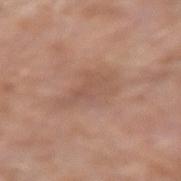No biopsy was performed on this lesion — it was imaged during a full skin examination and was not determined to be concerning.
On the arm.
Automated image analysis of the tile measured a shape eccentricity near 0.85 and a shape-asymmetry score of about 0.35 (0 = symmetric). And it measured border irregularity of about 4.5 on a 0–10 scale, internal color variation of about 3 on a 0–10 scale, and radial color variation of about 1. The software also gave a classifier nevus-likeness of about 0/100.
Imaged with white-light lighting.
A 15 mm crop from a total-body photograph taken for skin-cancer surveillance.
A male subject, aged 78–82.
The recorded lesion diameter is about 5.5 mm.